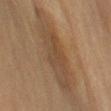workup: imaged on a skin check; not biopsied
acquisition: total-body-photography crop, ~15 mm field of view
location: the left upper arm
tile lighting: cross-polarized illumination
patient: female, aged around 70